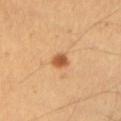<case>
<biopsy_status>not biopsied; imaged during a skin examination</biopsy_status>
<patient>
  <sex>male</sex>
  <age_approx>55</age_approx>
</patient>
<lighting>cross-polarized</lighting>
<site>left upper arm</site>
<image>
  <source>total-body photography crop</source>
  <field_of_view_mm>15</field_of_view_mm>
</image>
<automated_metrics>
  <area_mm2_approx>3.5</area_mm2_approx>
  <eccentricity>0.25</eccentricity>
  <shape_asymmetry>0.25</shape_asymmetry>
  <border_irregularity_0_10>2.0</border_irregularity_0_10>
  <color_variation_0_10>3.5</color_variation_0_10>
</automated_metrics>
<lesion_size>
  <long_diameter_mm_approx>2.0</long_diameter_mm_approx>
</lesion_size>
</case>